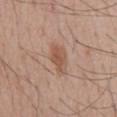Q: Was this lesion biopsied?
A: catalogued during a skin exam; not biopsied
Q: What is the lesion's diameter?
A: ≈3.5 mm
Q: What is the imaging modality?
A: ~15 mm tile from a whole-body skin photo
Q: What lighting was used for the tile?
A: white-light
Q: Patient demographics?
A: male, roughly 55 years of age
Q: What is the anatomic site?
A: the back